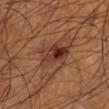Clinical impression:
The lesion was photographed on a routine skin check and not biopsied; there is no pathology result.
Context:
From the left lower leg. A male subject, aged around 55. A 15 mm crop from a total-body photograph taken for skin-cancer surveillance. Captured under cross-polarized illumination.